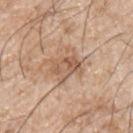biopsy status = imaged on a skin check; not biopsied
size = ~4 mm (longest diameter)
location = the right upper arm
automated metrics = a peripheral color-asymmetry measure near 2.5; a classifier nevus-likeness of about 0/100 and lesion-presence confidence of about 100/100
patient = male, aged approximately 65
imaging modality = ~15 mm tile from a whole-body skin photo
illumination = white-light illumination